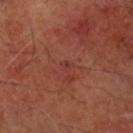follow-up: catalogued during a skin exam; not biopsied | diameter: ≈1.5 mm | lighting: cross-polarized | automated metrics: a lesion area of about 1.5 mm², a shape eccentricity near 0.25, and a symmetry-axis asymmetry near 0.35; roughly 4 lightness units darker than nearby skin and a lesion-to-skin contrast of about 4 (normalized; higher = more distinct); border irregularity of about 3 on a 0–10 scale, internal color variation of about 0 on a 0–10 scale, and radial color variation of about 0; a nevus-likeness score of about 5/100 and a detector confidence of about 100 out of 100 that the crop contains a lesion | site: the right forearm | patient: male, aged around 70 | image source: 15 mm crop, total-body photography.